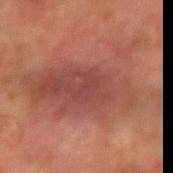The lesion was tiled from a total-body skin photograph and was not biopsied.
An algorithmic analysis of the crop reported a lesion area of about 60 mm² and a shape-asymmetry score of about 0.35 (0 = symmetric). And it measured an automated nevus-likeness rating near 5 out of 100 and a detector confidence of about 100 out of 100 that the crop contains a lesion.
A close-up tile cropped from a whole-body skin photograph, about 15 mm across.
About 13.5 mm across.
A male subject, aged around 60.
The tile uses cross-polarized illumination.
The lesion is on the left forearm.